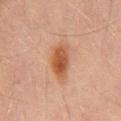Imaged during a routine full-body skin examination; the lesion was not biopsied and no histopathology is available.
Located on the mid back.
Measured at roughly 4.5 mm in maximum diameter.
A male patient roughly 45 years of age.
A roughly 15 mm field-of-view crop from a total-body skin photograph.